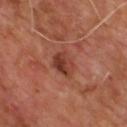Impression:
Imaged during a routine full-body skin examination; the lesion was not biopsied and no histopathology is available.
Context:
The lesion is located on the upper back. This is a cross-polarized tile. A close-up tile cropped from a whole-body skin photograph, about 15 mm across. About 3.5 mm across. A male patient in their mid-60s.